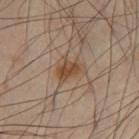workup: imaged on a skin check; not biopsied
tile lighting: cross-polarized
lesion diameter: ~3 mm (longest diameter)
location: the left thigh
automated metrics: a lesion area of about 6.5 mm², an eccentricity of roughly 0.6, and a shape-asymmetry score of about 0.45 (0 = symmetric); a lesion color around L≈47 a*≈17 b*≈30 in CIELAB and a lesion-to-skin contrast of about 8 (normalized; higher = more distinct); a classifier nevus-likeness of about 75/100
image source: 15 mm crop, total-body photography
patient: male, roughly 55 years of age